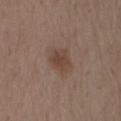This lesion was catalogued during total-body skin photography and was not selected for biopsy.
Cropped from a whole-body photographic skin survey; the tile spans about 15 mm.
Located on the lower back.
A male patient aged around 70.